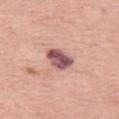Q: Was this lesion biopsied?
A: total-body-photography surveillance lesion; no biopsy
Q: Patient demographics?
A: female, aged approximately 65
Q: What is the imaging modality?
A: total-body-photography crop, ~15 mm field of view
Q: Where on the body is the lesion?
A: the left thigh